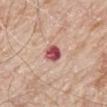Assessment: Imaged during a routine full-body skin examination; the lesion was not biopsied and no histopathology is available. Clinical summary: The total-body-photography lesion software estimated border irregularity of about 1.5 on a 0–10 scale and radial color variation of about 2. It also reported a classifier nevus-likeness of about 0/100. A roughly 15 mm field-of-view crop from a total-body skin photograph. The lesion is on the mid back. A male patient approximately 80 years of age. Imaged with white-light lighting.